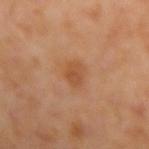follow-up: no biopsy performed (imaged during a skin exam) | TBP lesion metrics: a lesion area of about 5 mm², a shape eccentricity near 0.7, and a symmetry-axis asymmetry near 0.3; a lesion–skin lightness drop of about 7; a classifier nevus-likeness of about 30/100 and lesion-presence confidence of about 100/100 | subject: female, about 50 years old | body site: the right forearm | imaging modality: ~15 mm tile from a whole-body skin photo | lighting: cross-polarized illumination.